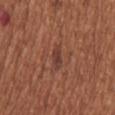Case summary:
– notes · total-body-photography surveillance lesion; no biopsy
– body site · the chest
– image source · total-body-photography crop, ~15 mm field of view
– subject · female, roughly 65 years of age